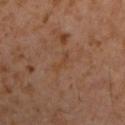Captured during whole-body skin photography for melanoma surveillance; the lesion was not biopsied.
The recorded lesion diameter is about 3 mm.
The total-body-photography lesion software estimated border irregularity of about 6.5 on a 0–10 scale, internal color variation of about 0 on a 0–10 scale, and radial color variation of about 0. The analysis additionally found a nevus-likeness score of about 0/100 and a lesion-detection confidence of about 95/100.
A 15 mm crop from a total-body photograph taken for skin-cancer surveillance.
This is a cross-polarized tile.
The lesion is located on the left upper arm.
A male subject, in their 60s.